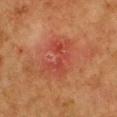No biopsy was performed on this lesion — it was imaged during a full skin examination and was not determined to be concerning. Imaged with cross-polarized lighting. From the front of the torso. Longest diameter approximately 4.5 mm. Automated tile analysis of the lesion measured a footprint of about 9.5 mm², an outline eccentricity of about 0.85 (0 = round, 1 = elongated), and a shape-asymmetry score of about 0.4 (0 = symmetric). The software also gave a mean CIELAB color near L≈38 a*≈29 b*≈28, about 6 CIELAB-L* units darker than the surrounding skin, and a normalized border contrast of about 5.5. It also reported a classifier nevus-likeness of about 0/100 and lesion-presence confidence of about 100/100. A close-up tile cropped from a whole-body skin photograph, about 15 mm across. A female subject in their 50s.